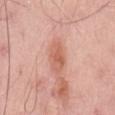Part of a total-body skin-imaging series; this lesion was reviewed on a skin check and was not flagged for biopsy. The lesion's longest dimension is about 4 mm. Located on the back. The subject is a male about 65 years old. A lesion tile, about 15 mm wide, cut from a 3D total-body photograph. Captured under white-light illumination. The lesion-visualizer software estimated a border-irregularity rating of about 2.5/10 and peripheral color asymmetry of about 1. It also reported an automated nevus-likeness rating near 0 out of 100 and a lesion-detection confidence of about 100/100.